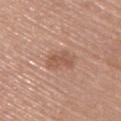follow-up = imaged on a skin check; not biopsied | lesion diameter = ≈3.5 mm | subject = female, aged around 65 | anatomic site = the upper back | acquisition = ~15 mm tile from a whole-body skin photo.